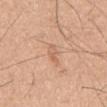| field | value |
|---|---|
| workup | catalogued during a skin exam; not biopsied |
| subject | male, aged approximately 45 |
| tile lighting | white-light illumination |
| size | about 2.5 mm |
| image source | ~15 mm tile from a whole-body skin photo |
| anatomic site | the mid back |
| image-analysis metrics | a symmetry-axis asymmetry near 0.35; a color-variation rating of about 0.5/10 and a peripheral color-asymmetry measure near 0 |